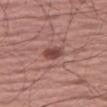This lesion was catalogued during total-body skin photography and was not selected for biopsy.
The tile uses white-light illumination.
Cropped from a whole-body photographic skin survey; the tile spans about 15 mm.
The patient is a male aged approximately 65.
On the right thigh.
Measured at roughly 2.5 mm in maximum diameter.